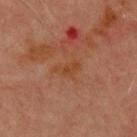The lesion was photographed on a routine skin check and not biopsied; there is no pathology result. Longest diameter approximately 3 mm. An algorithmic analysis of the crop reported an average lesion color of about L≈35 a*≈20 b*≈30 (CIELAB), a lesion–skin lightness drop of about 5, and a normalized border contrast of about 6. A lesion tile, about 15 mm wide, cut from a 3D total-body photograph. The subject is a male in their 70s. The tile uses cross-polarized illumination. The lesion is on the chest.